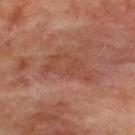{"biopsy_status": "not biopsied; imaged during a skin examination", "lighting": "cross-polarized", "image": {"source": "total-body photography crop", "field_of_view_mm": 15}, "automated_metrics": {"eccentricity": 0.9, "shape_asymmetry": 0.45, "border_irregularity_0_10": 5.5, "color_variation_0_10": 2.5, "peripheral_color_asymmetry": 1.0, "nevus_likeness_0_100": 0}, "patient": {"sex": "male", "age_approx": 65}, "lesion_size": {"long_diameter_mm_approx": 6.0}, "site": "chest"}